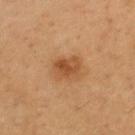Assessment:
This lesion was catalogued during total-body skin photography and was not selected for biopsy.
Clinical summary:
A male patient, in their 60s. The lesion's longest dimension is about 3 mm. A 15 mm crop from a total-body photograph taken for skin-cancer surveillance. This is a cross-polarized tile.